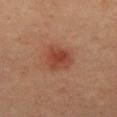The lesion was tiled from a total-body skin photograph and was not biopsied.
Located on the mid back.
This image is a 15 mm lesion crop taken from a total-body photograph.
Automated image analysis of the tile measured a footprint of about 8.5 mm², an eccentricity of roughly 0.55, and a symmetry-axis asymmetry near 0.3. It also reported a border-irregularity index near 3/10, a color-variation rating of about 3/10, and a peripheral color-asymmetry measure near 1. The analysis additionally found a lesion-detection confidence of about 100/100.
A male subject, aged 58 to 62.
About 3.5 mm across.
Imaged with cross-polarized lighting.